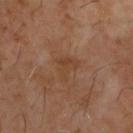- image-analysis metrics — a border-irregularity rating of about 5.5/10, a color-variation rating of about 2/10, and a peripheral color-asymmetry measure near 0.5; a nevus-likeness score of about 0/100 and a detector confidence of about 100 out of 100 that the crop contains a lesion
- image — total-body-photography crop, ~15 mm field of view
- anatomic site — the upper back
- subject — male, aged around 60